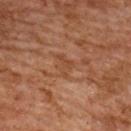– image — ~15 mm crop, total-body skin-cancer survey
– patient — female, about 60 years old
– location — the upper back
– lesion size — about 3 mm
– tile lighting — cross-polarized